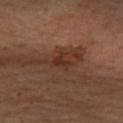Part of a total-body skin-imaging series; this lesion was reviewed on a skin check and was not flagged for biopsy. A female patient, aged approximately 40. The lesion is located on the left forearm. A region of skin cropped from a whole-body photographic capture, roughly 15 mm wide. The tile uses cross-polarized illumination.